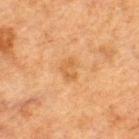No biopsy was performed on this lesion — it was imaged during a full skin examination and was not determined to be concerning. A lesion tile, about 15 mm wide, cut from a 3D total-body photograph. The patient is a male about 50 years old. On the upper back. The recorded lesion diameter is about 3 mm. This is a cross-polarized tile. Automated image analysis of the tile measured an average lesion color of about L≈48 a*≈19 b*≈36 (CIELAB) and a lesion-to-skin contrast of about 5 (normalized; higher = more distinct). The analysis additionally found a border-irregularity rating of about 3.5/10, internal color variation of about 2 on a 0–10 scale, and a peripheral color-asymmetry measure near 0.5.